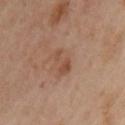The lesion's longest dimension is about 3.5 mm. A female patient, approximately 40 years of age. This image is a 15 mm lesion crop taken from a total-body photograph. An algorithmic analysis of the crop reported a footprint of about 4.5 mm², an outline eccentricity of about 0.9 (0 = round, 1 = elongated), and a shape-asymmetry score of about 0.45 (0 = symmetric). The software also gave a classifier nevus-likeness of about 5/100 and a detector confidence of about 100 out of 100 that the crop contains a lesion. The lesion is located on the left upper arm. The tile uses cross-polarized illumination.